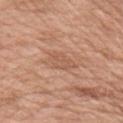The lesion was tiled from a total-body skin photograph and was not biopsied. Automated image analysis of the tile measured an average lesion color of about L≈57 a*≈22 b*≈32 (CIELAB), roughly 7 lightness units darker than nearby skin, and a lesion-to-skin contrast of about 4.5 (normalized; higher = more distinct). It also reported a border-irregularity rating of about 3/10, internal color variation of about 2 on a 0–10 scale, and peripheral color asymmetry of about 0.5. The analysis additionally found an automated nevus-likeness rating near 0 out of 100 and lesion-presence confidence of about 95/100. The lesion is located on the right upper arm. This is a white-light tile. A close-up tile cropped from a whole-body skin photograph, about 15 mm across. A female subject, approximately 55 years of age.